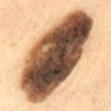The lesion was photographed on a routine skin check and not biopsied; there is no pathology result.
A male subject about 60 years old.
Located on the abdomen.
A close-up tile cropped from a whole-body skin photograph, about 15 mm across.